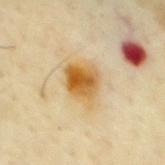Recorded during total-body skin imaging; not selected for excision or biopsy. The patient is a male aged around 65. A close-up tile cropped from a whole-body skin photograph, about 15 mm across. The tile uses cross-polarized illumination. Automated tile analysis of the lesion measured an area of roughly 11 mm² and a shape eccentricity near 0.55. The lesion is located on the front of the torso.